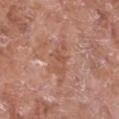notes: no biopsy performed (imaged during a skin exam) | image source: ~15 mm crop, total-body skin-cancer survey | anatomic site: the chest | patient: female, aged 73 to 77.